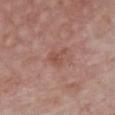Q: Was this lesion biopsied?
A: imaged on a skin check; not biopsied
Q: Automated lesion metrics?
A: internal color variation of about 3 on a 0–10 scale and peripheral color asymmetry of about 1.5; an automated nevus-likeness rating near 0 out of 100 and a lesion-detection confidence of about 100/100
Q: What is the anatomic site?
A: the chest
Q: Who is the patient?
A: male, aged approximately 85 or older
Q: Lesion size?
A: ≈2.5 mm
Q: What lighting was used for the tile?
A: white-light illumination
Q: What is the imaging modality?
A: ~15 mm crop, total-body skin-cancer survey